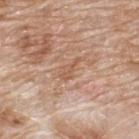Imaged during a routine full-body skin examination; the lesion was not biopsied and no histopathology is available.
A 15 mm close-up tile from a total-body photography series done for melanoma screening.
A male patient, about 80 years old.
On the upper back.
The tile uses white-light illumination.